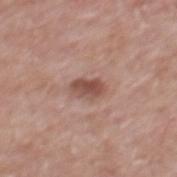<case>
<lighting>white-light</lighting>
<site>mid back</site>
<lesion_size>
  <long_diameter_mm_approx>3.0</long_diameter_mm_approx>
</lesion_size>
<patient>
  <sex>male</sex>
  <age_approx>70</age_approx>
</patient>
<automated_metrics>
  <area_mm2_approx>4.5</area_mm2_approx>
  <eccentricity>0.8</eccentricity>
  <border_irregularity_0_10>2.5</border_irregularity_0_10>
  <color_variation_0_10>3.5</color_variation_0_10>
  <peripheral_color_asymmetry>1.5</peripheral_color_asymmetry>
  <nevus_likeness_0_100>85</nevus_likeness_0_100>
  <lesion_detection_confidence_0_100>100</lesion_detection_confidence_0_100>
</automated_metrics>
<image>
  <source>total-body photography crop</source>
  <field_of_view_mm>15</field_of_view_mm>
</image>
</case>